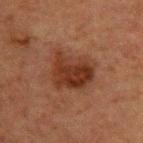| key | value |
|---|---|
| workup | imaged on a skin check; not biopsied |
| anatomic site | the back |
| image | ~15 mm crop, total-body skin-cancer survey |
| lesion size | about 5 mm |
| subject | male, aged approximately 50 |
| TBP lesion metrics | a lesion–skin lightness drop of about 8 and a normalized lesion–skin contrast near 8.5; border irregularity of about 2.5 on a 0–10 scale, internal color variation of about 4.5 on a 0–10 scale, and radial color variation of about 1.5; a nevus-likeness score of about 60/100 and a lesion-detection confidence of about 100/100 |
| tile lighting | cross-polarized illumination |